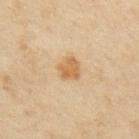This lesion was catalogued during total-body skin photography and was not selected for biopsy.
Captured under cross-polarized illumination.
A close-up tile cropped from a whole-body skin photograph, about 15 mm across.
The lesion is located on the upper back.
Measured at roughly 2.5 mm in maximum diameter.
The subject is a female roughly 35 years of age.
An algorithmic analysis of the crop reported border irregularity of about 2.5 on a 0–10 scale and a within-lesion color-variation index near 2.5/10.